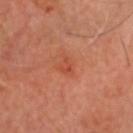<lesion>
<image>
  <source>total-body photography crop</source>
  <field_of_view_mm>15</field_of_view_mm>
</image>
<lesion_size>
  <long_diameter_mm_approx>3.0</long_diameter_mm_approx>
</lesion_size>
<patient>
  <sex>male</sex>
  <age_approx>70</age_approx>
</patient>
<automated_metrics>
  <area_mm2_approx>3.5</area_mm2_approx>
  <cielab_L>48</cielab_L>
  <cielab_a>31</cielab_a>
  <cielab_b>34</cielab_b>
  <vs_skin_darker_L>6.0</vs_skin_darker_L>
  <vs_skin_contrast_norm>5.0</vs_skin_contrast_norm>
  <color_variation_0_10>2.0</color_variation_0_10>
  <peripheral_color_asymmetry>0.5</peripheral_color_asymmetry>
  <nevus_likeness_0_100>0</nevus_likeness_0_100>
  <lesion_detection_confidence_0_100>100</lesion_detection_confidence_0_100>
</automated_metrics>
<site>head or neck</site>
<lighting>cross-polarized</lighting>
</lesion>